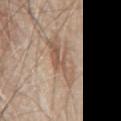Measured at roughly 5.5 mm in maximum diameter. Automated image analysis of the tile measured a footprint of about 9 mm², an outline eccentricity of about 0.9 (0 = round, 1 = elongated), and a symmetry-axis asymmetry near 0.35. The lesion is on the mid back. This image is a 15 mm lesion crop taken from a total-body photograph. This is a white-light tile. The patient is a male aged 78–82.